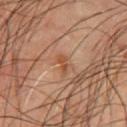Impression:
Imaged during a routine full-body skin examination; the lesion was not biopsied and no histopathology is available.
Context:
This image is a 15 mm lesion crop taken from a total-body photograph. The lesion's longest dimension is about 2.5 mm. The patient is a male in their mid-60s. The tile uses cross-polarized illumination. On the chest.